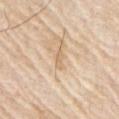Q: Was this lesion biopsied?
A: imaged on a skin check; not biopsied
Q: What is the lesion's diameter?
A: ≈2.5 mm
Q: What did automated image analysis measure?
A: a mean CIELAB color near L≈69 a*≈15 b*≈35, about 7 CIELAB-L* units darker than the surrounding skin, and a normalized lesion–skin contrast near 5; a classifier nevus-likeness of about 0/100
Q: What is the anatomic site?
A: the right upper arm
Q: What are the patient's age and sex?
A: male, roughly 80 years of age
Q: How was this image acquired?
A: 15 mm crop, total-body photography